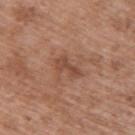A male subject, in their 50s. A lesion tile, about 15 mm wide, cut from a 3D total-body photograph. The lesion is on the back. The lesion's longest dimension is about 3 mm. Imaged with white-light lighting.